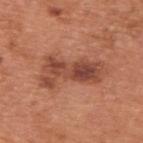The lesion was photographed on a routine skin check and not biopsied; there is no pathology result. A male patient, aged around 65. On the upper back. A roughly 15 mm field-of-view crop from a total-body skin photograph.